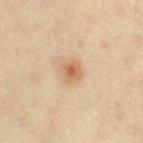Clinical impression: The lesion was photographed on a routine skin check and not biopsied; there is no pathology result. Clinical summary: A female subject, roughly 45 years of age. Cropped from a total-body skin-imaging series; the visible field is about 15 mm. From the leg.